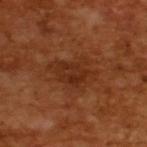The lesion was photographed on a routine skin check and not biopsied; there is no pathology result.
A 15 mm close-up extracted from a 3D total-body photography capture.
This is a cross-polarized tile.
The patient is a male about 65 years old.
The total-body-photography lesion software estimated a shape eccentricity near 0.7 and a symmetry-axis asymmetry near 0.3. The analysis additionally found a mean CIELAB color near L≈30 a*≈24 b*≈32 and a lesion-to-skin contrast of about 6 (normalized; higher = more distinct).